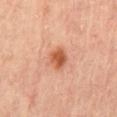Notes:
• notes: catalogued during a skin exam; not biopsied
• automated metrics: an area of roughly 5 mm², an eccentricity of roughly 0.45, and a shape-asymmetry score of about 0.15 (0 = symmetric); peripheral color asymmetry of about 1
• subject: male, aged 63 to 67
• body site: the lower back
• image: total-body-photography crop, ~15 mm field of view
• illumination: cross-polarized